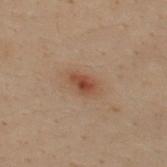No biopsy was performed on this lesion — it was imaged during a full skin examination and was not determined to be concerning. Approximately 3 mm at its widest. The lesion is on the upper back. A 15 mm close-up extracted from a 3D total-body photography capture. The patient is a male aged 28–32.